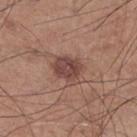No biopsy was performed on this lesion — it was imaged during a full skin examination and was not determined to be concerning. The recorded lesion diameter is about 4 mm. Cropped from a whole-body photographic skin survey; the tile spans about 15 mm. A male subject, aged around 60. Imaged with white-light lighting. On the left lower leg.